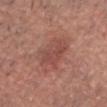{
  "biopsy_status": "not biopsied; imaged during a skin examination",
  "patient": {
    "sex": "male",
    "age_approx": 45
  },
  "image": {
    "source": "total-body photography crop",
    "field_of_view_mm": 15
  },
  "site": "head or neck",
  "lesion_size": {
    "long_diameter_mm_approx": 4.5
  }
}